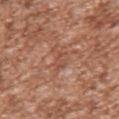anatomic site — the left upper arm | patient — male, aged approximately 45 | TBP lesion metrics — a detector confidence of about 80 out of 100 that the crop contains a lesion | image — total-body-photography crop, ~15 mm field of view | diameter — ≈3 mm.